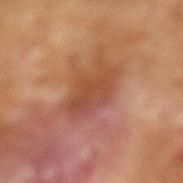biopsy status = total-body-photography surveillance lesion; no biopsy
patient = male, aged 63–67
automated metrics = a lesion area of about 14 mm², an eccentricity of roughly 0.75, and two-axis asymmetry of about 0.3; border irregularity of about 3.5 on a 0–10 scale, a within-lesion color-variation index near 5/10, and a peripheral color-asymmetry measure near 2
image = ~15 mm crop, total-body skin-cancer survey
lesion size = ≈5.5 mm